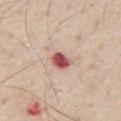Findings:
- biopsy status: no biopsy performed (imaged during a skin exam)
- imaging modality: ~15 mm tile from a whole-body skin photo
- subject: male, aged 78 to 82
- site: the chest